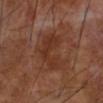The lesion was photographed on a routine skin check and not biopsied; there is no pathology result. On the left forearm. Cropped from a total-body skin-imaging series; the visible field is about 15 mm. The lesion's longest dimension is about 6 mm. A male subject, aged 68–72. The tile uses cross-polarized illumination.